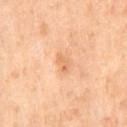Background: The subject is a female aged around 55. Imaged with cross-polarized lighting. The lesion is located on the right thigh. Measured at roughly 3 mm in maximum diameter. A close-up tile cropped from a whole-body skin photograph, about 15 mm across.